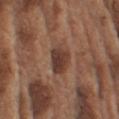Findings:
- acquisition · 15 mm crop, total-body photography
- automated metrics · a footprint of about 7.5 mm², a shape eccentricity near 0.55, and a symmetry-axis asymmetry near 0.2; a lesion color around L≈38 a*≈19 b*≈25 in CIELAB, roughly 11 lightness units darker than nearby skin, and a lesion-to-skin contrast of about 9.5 (normalized; higher = more distinct); a peripheral color-asymmetry measure near 1; a nevus-likeness score of about 60/100
- lesion diameter · ~3.5 mm (longest diameter)
- illumination · white-light
- patient · male, roughly 75 years of age
- body site · the left upper arm